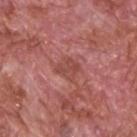This lesion was catalogued during total-body skin photography and was not selected for biopsy. A close-up tile cropped from a whole-body skin photograph, about 15 mm across. The lesion is located on the head or neck. The subject is a male about 60 years old.